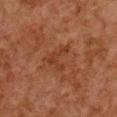<tbp_lesion>
  <site>chest</site>
  <lighting>cross-polarized</lighting>
  <image>
    <source>total-body photography crop</source>
    <field_of_view_mm>15</field_of_view_mm>
  </image>
  <lesion_size>
    <long_diameter_mm_approx>3.5</long_diameter_mm_approx>
  </lesion_size>
  <patient>
    <sex>female</sex>
    <age_approx>60</age_approx>
  </patient>
  <automated_metrics>
    <cielab_L>34</cielab_L>
    <cielab_a>23</cielab_a>
    <cielab_b>31</cielab_b>
    <vs_skin_darker_L>5.0</vs_skin_darker_L>
    <vs_skin_contrast_norm>5.5</vs_skin_contrast_norm>
    <nevus_likeness_0_100>0</nevus_likeness_0_100>
    <lesion_detection_confidence_0_100>100</lesion_detection_confidence_0_100>
  </automated_metrics>
</tbp_lesion>